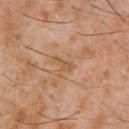The lesion is located on the chest.
A 15 mm close-up extracted from a 3D total-body photography capture.
A male subject aged around 60.
This is a cross-polarized tile.
The lesion-visualizer software estimated an area of roughly 2.5 mm², an eccentricity of roughly 0.9, and a symmetry-axis asymmetry near 0.55. It also reported a classifier nevus-likeness of about 0/100.